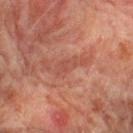Q: Is there a histopathology result?
A: no biopsy performed (imaged during a skin exam)
Q: What kind of image is this?
A: total-body-photography crop, ~15 mm field of view
Q: What is the lesion's diameter?
A: ~3 mm (longest diameter)
Q: Patient demographics?
A: male, aged approximately 75
Q: Lesion location?
A: the leg